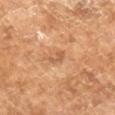Q: Was a biopsy performed?
A: catalogued during a skin exam; not biopsied
Q: How was this image acquired?
A: ~15 mm crop, total-body skin-cancer survey
Q: What lighting was used for the tile?
A: cross-polarized illumination
Q: Patient demographics?
A: male, aged 63–67